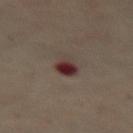The lesion was tiled from a total-body skin photograph and was not biopsied. A lesion tile, about 15 mm wide, cut from a 3D total-body photograph. About 3.5 mm across. The lesion is on the front of the torso. The patient is a female aged 58 to 62. The total-body-photography lesion software estimated a footprint of about 5.5 mm² and a symmetry-axis asymmetry near 0.2. The analysis additionally found a border-irregularity rating of about 2/10, a color-variation rating of about 9.5/10, and peripheral color asymmetry of about 3.5. Imaged with cross-polarized lighting.